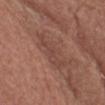biopsy status: imaged on a skin check; not biopsied | site: the chest | subject: female, about 65 years old | image source: ~15 mm crop, total-body skin-cancer survey | automated lesion analysis: a lesion area of about 7 mm², an outline eccentricity of about 0.95 (0 = round, 1 = elongated), and two-axis asymmetry of about 0.55; roughly 6 lightness units darker than nearby skin and a normalized lesion–skin contrast near 4.5; a border-irregularity rating of about 7/10 and peripheral color asymmetry of about 0.5; a nevus-likeness score of about 0/100 and a detector confidence of about 80 out of 100 that the crop contains a lesion | illumination: white-light | size: ~5 mm (longest diameter).